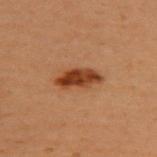No biopsy was performed on this lesion — it was imaged during a full skin examination and was not determined to be concerning.
A female subject, in their 50s.
Cropped from a whole-body photographic skin survey; the tile spans about 15 mm.
On the upper back.